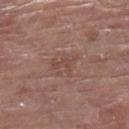The lesion was tiled from a total-body skin photograph and was not biopsied. An algorithmic analysis of the crop reported an area of roughly 4 mm² and an outline eccentricity of about 0.85 (0 = round, 1 = elongated). The software also gave a nevus-likeness score of about 0/100 and lesion-presence confidence of about 100/100. The subject is a female in their 80s. From the leg. A close-up tile cropped from a whole-body skin photograph, about 15 mm across.